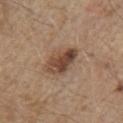biopsy status = no biopsy performed (imaged during a skin exam) | subject = male, roughly 70 years of age | tile lighting = white-light illumination | acquisition = ~15 mm crop, total-body skin-cancer survey | site = the chest.